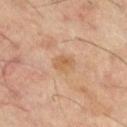No biopsy was performed on this lesion — it was imaged during a full skin examination and was not determined to be concerning. The lesion is located on the right thigh. Automated tile analysis of the lesion measured an average lesion color of about L≈55 a*≈18 b*≈35 (CIELAB), roughly 7 lightness units darker than nearby skin, and a lesion-to-skin contrast of about 6 (normalized; higher = more distinct). The analysis additionally found a border-irregularity index near 2.5/10 and a color-variation rating of about 2.5/10. A 15 mm crop from a total-body photograph taken for skin-cancer surveillance. The patient is a male aged around 60. Imaged with cross-polarized lighting. Measured at roughly 2.5 mm in maximum diameter.